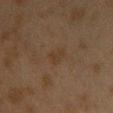{
  "biopsy_status": "not biopsied; imaged during a skin examination",
  "automated_metrics": {
    "nevus_likeness_0_100": 10,
    "lesion_detection_confidence_0_100": 100
  },
  "site": "chest",
  "lighting": "cross-polarized",
  "lesion_size": {
    "long_diameter_mm_approx": 2.5
  },
  "patient": {
    "sex": "female",
    "age_approx": 40
  },
  "image": {
    "source": "total-body photography crop",
    "field_of_view_mm": 15
  }
}